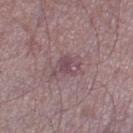<record>
  <biopsy_status>not biopsied; imaged during a skin examination</biopsy_status>
  <patient>
    <sex>male</sex>
    <age_approx>65</age_approx>
  </patient>
  <automated_metrics>
    <cielab_L>47</cielab_L>
    <cielab_a>19</cielab_a>
    <cielab_b>13</cielab_b>
    <vs_skin_contrast_norm>6.0</vs_skin_contrast_norm>
    <border_irregularity_0_10>5.5</border_irregularity_0_10>
    <color_variation_0_10>2.5</color_variation_0_10>
    <peripheral_color_asymmetry>1.0</peripheral_color_asymmetry>
  </automated_metrics>
  <lighting>white-light</lighting>
  <site>left lower leg</site>
  <image>
    <source>total-body photography crop</source>
    <field_of_view_mm>15</field_of_view_mm>
  </image>
</record>